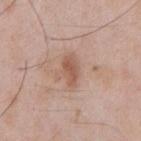follow-up: total-body-photography surveillance lesion; no biopsy
tile lighting: white-light illumination
subject: male, aged 63–67
location: the chest
image source: ~15 mm crop, total-body skin-cancer survey
lesion diameter: ≈3.5 mm
automated lesion analysis: about 10 CIELAB-L* units darker than the surrounding skin and a normalized border contrast of about 7; a border-irregularity rating of about 2/10, internal color variation of about 2.5 on a 0–10 scale, and radial color variation of about 1; a classifier nevus-likeness of about 10/100 and a lesion-detection confidence of about 100/100